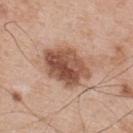On the upper back. Approximately 6 mm at its widest. This is a white-light tile. A lesion tile, about 15 mm wide, cut from a 3D total-body photograph. Automated tile analysis of the lesion measured a mean CIELAB color near L≈53 a*≈22 b*≈29, about 15 CIELAB-L* units darker than the surrounding skin, and a normalized lesion–skin contrast near 10. The patient is a male aged approximately 55.